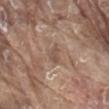<record>
<biopsy_status>not biopsied; imaged during a skin examination</biopsy_status>
<lighting>white-light</lighting>
<image>
  <source>total-body photography crop</source>
  <field_of_view_mm>15</field_of_view_mm>
</image>
<patient>
  <sex>male</sex>
  <age_approx>80</age_approx>
</patient>
<site>mid back</site>
<automated_metrics>
  <cielab_L>51</cielab_L>
  <cielab_a>16</cielab_a>
  <cielab_b>26</cielab_b>
  <vs_skin_darker_L>7.0</vs_skin_darker_L>
  <vs_skin_contrast_norm>5.5</vs_skin_contrast_norm>
  <nevus_likeness_0_100>0</nevus_likeness_0_100>
  <lesion_detection_confidence_0_100>90</lesion_detection_confidence_0_100>
</automated_metrics>
</record>